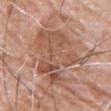A close-up tile cropped from a whole-body skin photograph, about 15 mm across.
On the back.
A male patient, aged 63–67.
About 9 mm across.